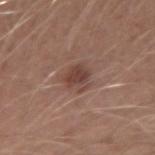Case summary:
• anatomic site — the left forearm
• automated lesion analysis — an area of roughly 6.5 mm² and a symmetry-axis asymmetry near 0.3; a lesion color around L≈43 a*≈19 b*≈24 in CIELAB, roughly 9 lightness units darker than nearby skin, and a normalized lesion–skin contrast near 7; a border-irregularity rating of about 3/10, a within-lesion color-variation index near 3/10, and radial color variation of about 1
• subject — male, aged around 25
• acquisition — ~15 mm crop, total-body skin-cancer survey